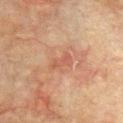Q: Was this lesion biopsied?
A: total-body-photography surveillance lesion; no biopsy
Q: Where on the body is the lesion?
A: the chest
Q: What are the patient's age and sex?
A: male, in their mid- to late 70s
Q: What is the lesion's diameter?
A: ~3 mm (longest diameter)
Q: What is the imaging modality?
A: 15 mm crop, total-body photography